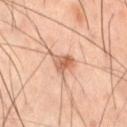<record>
<biopsy_status>not biopsied; imaged during a skin examination</biopsy_status>
<patient>
  <sex>male</sex>
  <age_approx>60</age_approx>
</patient>
<image>
  <source>total-body photography crop</source>
  <field_of_view_mm>15</field_of_view_mm>
</image>
<lighting>cross-polarized</lighting>
<site>right thigh</site>
<automated_metrics>
  <eccentricity>0.5</eccentricity>
  <shape_asymmetry>0.4</shape_asymmetry>
  <cielab_L>62</cielab_L>
  <cielab_a>22</cielab_a>
  <cielab_b>31</cielab_b>
  <vs_skin_darker_L>11.0</vs_skin_darker_L>
  <vs_skin_contrast_norm>7.0</vs_skin_contrast_norm>
  <border_irregularity_0_10>5.0</border_irregularity_0_10>
  <peripheral_color_asymmetry>2.5</peripheral_color_asymmetry>
  <lesion_detection_confidence_0_100>100</lesion_detection_confidence_0_100>
</automated_metrics>
</record>